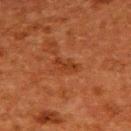The lesion was photographed on a routine skin check and not biopsied; there is no pathology result. The lesion's longest dimension is about 3 mm. The tile uses cross-polarized illumination. A female subject, aged 48–52. A roughly 15 mm field-of-view crop from a total-body skin photograph. Located on the upper back.